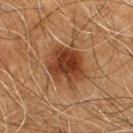Part of a total-body skin-imaging series; this lesion was reviewed on a skin check and was not flagged for biopsy.